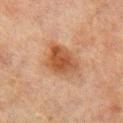Part of a total-body skin-imaging series; this lesion was reviewed on a skin check and was not flagged for biopsy.
Longest diameter approximately 5 mm.
A 15 mm crop from a total-body photograph taken for skin-cancer surveillance.
A male subject, aged 68–72.
Captured under cross-polarized illumination.
The lesion is on the arm.
The lesion-visualizer software estimated border irregularity of about 2.5 on a 0–10 scale, a color-variation rating of about 5/10, and peripheral color asymmetry of about 1.5. And it measured a nevus-likeness score of about 95/100 and lesion-presence confidence of about 100/100.